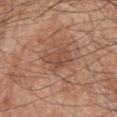Imaged during a routine full-body skin examination; the lesion was not biopsied and no histopathology is available.
The lesion is located on the upper back.
A 15 mm close-up extracted from a 3D total-body photography capture.
A male patient, approximately 45 years of age.
Approximately 4.5 mm at its widest.